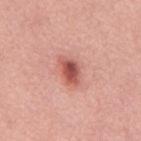| key | value |
|---|---|
| image | 15 mm crop, total-body photography |
| lighting | white-light illumination |
| size | ~3.5 mm (longest diameter) |
| patient | male, aged 58 to 62 |
| anatomic site | the back |